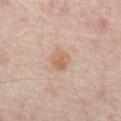{
  "biopsy_status": "not biopsied; imaged during a skin examination",
  "site": "abdomen",
  "automated_metrics": {
    "area_mm2_approx": 5.5,
    "eccentricity": 0.55,
    "shape_asymmetry": 0.2,
    "cielab_L": 61,
    "cielab_a": 19,
    "cielab_b": 30,
    "vs_skin_darker_L": 8.0,
    "border_irregularity_0_10": 2.0,
    "peripheral_color_asymmetry": 1.0
  },
  "image": {
    "source": "total-body photography crop",
    "field_of_view_mm": 15
  },
  "patient": {
    "age_approx": 55
  }
}